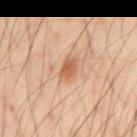notes: catalogued during a skin exam; not biopsied | subject: male, aged 53 to 57 | automated metrics: an area of roughly 4 mm² and a shape-asymmetry score of about 0.2 (0 = symmetric); a nevus-likeness score of about 90/100 | location: the mid back | image: 15 mm crop, total-body photography | illumination: cross-polarized | size: ≈2.5 mm.